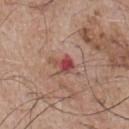Recorded during total-body skin imaging; not selected for excision or biopsy.